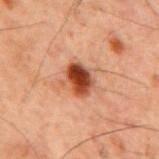Notes:
* follow-up — imaged on a skin check; not biopsied
* image-analysis metrics — a border-irregularity index near 1.5/10, a color-variation rating of about 7/10, and radial color variation of about 2; a classifier nevus-likeness of about 100/100 and a lesion-detection confidence of about 100/100
* subject — male, aged 58–62
* lesion diameter — about 3.5 mm
* image — ~15 mm crop, total-body skin-cancer survey
* site — the mid back
* lighting — cross-polarized illumination